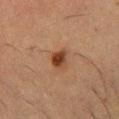notes: imaged on a skin check; not biopsied | automated lesion analysis: a classifier nevus-likeness of about 100/100 and a detector confidence of about 100 out of 100 that the crop contains a lesion | imaging modality: ~15 mm crop, total-body skin-cancer survey | patient: male, approximately 50 years of age | body site: the leg.